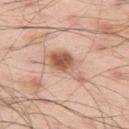Q: Was this lesion biopsied?
A: no biopsy performed (imaged during a skin exam)
Q: What kind of image is this?
A: ~15 mm tile from a whole-body skin photo
Q: Automated lesion metrics?
A: an area of roughly 10 mm², an outline eccentricity of about 0.85 (0 = round, 1 = elongated), and a shape-asymmetry score of about 0.45 (0 = symmetric); an average lesion color of about L≈60 a*≈21 b*≈30 (CIELAB) and a normalized lesion–skin contrast near 8; a classifier nevus-likeness of about 95/100 and a lesion-detection confidence of about 100/100
Q: Illumination type?
A: white-light
Q: What are the patient's age and sex?
A: male, aged 53–57
Q: What is the anatomic site?
A: the left thigh
Q: What is the lesion's diameter?
A: about 5.5 mm